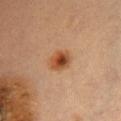Recorded during total-body skin imaging; not selected for excision or biopsy.
The lesion's longest dimension is about 3 mm.
A region of skin cropped from a whole-body photographic capture, roughly 15 mm wide.
The lesion is located on the chest.
The tile uses cross-polarized illumination.
A female patient, about 60 years old.